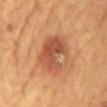<lesion>
  <biopsy_status>not biopsied; imaged during a skin examination</biopsy_status>
  <image>
    <source>total-body photography crop</source>
    <field_of_view_mm>15</field_of_view_mm>
  </image>
  <site>chest</site>
  <lighting>cross-polarized</lighting>
  <lesion_size>
    <long_diameter_mm_approx>5.0</long_diameter_mm_approx>
  </lesion_size>
  <patient>
    <sex>male</sex>
    <age_approx>85+</age_approx>
  </patient>
</lesion>